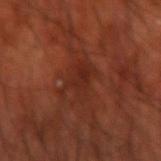site: the right forearm | tile lighting: cross-polarized | imaging modality: ~15 mm crop, total-body skin-cancer survey | subject: male, roughly 70 years of age.